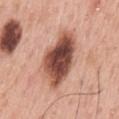Impression:
The lesion was tiled from a total-body skin photograph and was not biopsied.
Background:
A male patient, about 60 years old. The lesion is located on the back. Captured under white-light illumination. The lesion's longest dimension is about 7 mm. Cropped from a total-body skin-imaging series; the visible field is about 15 mm.